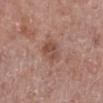The lesion was tiled from a total-body skin photograph and was not biopsied. Imaged with white-light lighting. A close-up tile cropped from a whole-body skin photograph, about 15 mm across. The lesion is on the left lower leg. Measured at roughly 3 mm in maximum diameter. A female patient, about 65 years old.